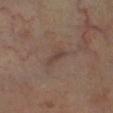Notes:
• follow-up · no biopsy performed (imaged during a skin exam)
• patient · male, aged 58–62
• anatomic site · the right lower leg
• image · ~15 mm tile from a whole-body skin photo